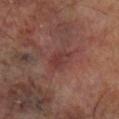Case summary:
* follow-up: catalogued during a skin exam; not biopsied
* anatomic site: the leg
* patient: male, approximately 70 years of age
* tile lighting: cross-polarized
* image source: total-body-photography crop, ~15 mm field of view
* image-analysis metrics: a footprint of about 5.5 mm², a shape eccentricity near 0.65, and a symmetry-axis asymmetry near 0.25; an average lesion color of about L≈36 a*≈23 b*≈21 (CIELAB), a lesion–skin lightness drop of about 6, and a lesion-to-skin contrast of about 5.5 (normalized; higher = more distinct)
* lesion diameter: ≈3 mm